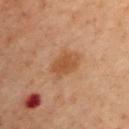Clinical impression:
No biopsy was performed on this lesion — it was imaged during a full skin examination and was not determined to be concerning.
Clinical summary:
The lesion is on the right upper arm. A close-up tile cropped from a whole-body skin photograph, about 15 mm across. About 3.5 mm across. The tile uses cross-polarized illumination. The patient is a male approximately 60 years of age.